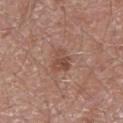{
  "biopsy_status": "not biopsied; imaged during a skin examination",
  "site": "left lower leg",
  "lesion_size": {
    "long_diameter_mm_approx": 2.5
  },
  "patient": {
    "sex": "male",
    "age_approx": 60
  },
  "image": {
    "source": "total-body photography crop",
    "field_of_view_mm": 15
  }
}